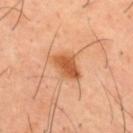Assessment:
Part of a total-body skin-imaging series; this lesion was reviewed on a skin check and was not flagged for biopsy.
Background:
This image is a 15 mm lesion crop taken from a total-body photograph. The subject is a male aged approximately 40. On the mid back. Automated image analysis of the tile measured an outline eccentricity of about 0.7 (0 = round, 1 = elongated). The analysis additionally found a border-irregularity rating of about 2.5/10, a within-lesion color-variation index near 4.5/10, and radial color variation of about 1.5. And it measured a classifier nevus-likeness of about 90/100.